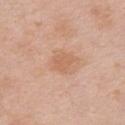{"biopsy_status": "not biopsied; imaged during a skin examination", "image": {"source": "total-body photography crop", "field_of_view_mm": 15}, "site": "front of the torso", "lesion_size": {"long_diameter_mm_approx": 2.5}, "lighting": "white-light", "patient": {"sex": "female", "age_approx": 50}}